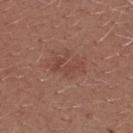follow-up: no biopsy performed (imaged during a skin exam)
site: the upper back
TBP lesion metrics: a detector confidence of about 100 out of 100 that the crop contains a lesion
image source: ~15 mm tile from a whole-body skin photo
lesion diameter: ~4 mm (longest diameter)
subject: female, about 25 years old